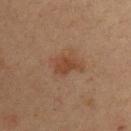{"biopsy_status": "not biopsied; imaged during a skin examination", "image": {"source": "total-body photography crop", "field_of_view_mm": 15}, "patient": {"sex": "male", "age_approx": 35}, "lighting": "cross-polarized", "site": "chest", "lesion_size": {"long_diameter_mm_approx": 3.0}}